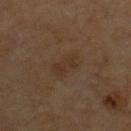follow-up: catalogued during a skin exam; not biopsied
acquisition: ~15 mm tile from a whole-body skin photo
automated lesion analysis: a mean CIELAB color near L≈29 a*≈13 b*≈24, roughly 4 lightness units darker than nearby skin, and a lesion-to-skin contrast of about 5 (normalized; higher = more distinct); a border-irregularity index near 2.5/10 and a color-variation rating of about 2.5/10
lighting: cross-polarized
subject: male, approximately 60 years of age
body site: the chest
lesion diameter: ~3.5 mm (longest diameter)